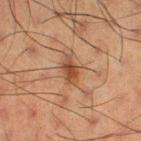From the right thigh. The total-body-photography lesion software estimated a border-irregularity index near 3/10 and radial color variation of about 1. A male subject, aged around 60. A region of skin cropped from a whole-body photographic capture, roughly 15 mm wide.